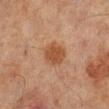workup — imaged on a skin check; not biopsied
subject — male, aged 63 to 67
image-analysis metrics — an area of roughly 7.5 mm², a shape eccentricity near 0.55, and two-axis asymmetry of about 0.2; a lesion–skin lightness drop of about 8 and a normalized border contrast of about 8
imaging modality — ~15 mm tile from a whole-body skin photo
illumination — cross-polarized
anatomic site — the right lower leg